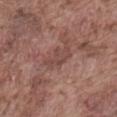Q: Is there a histopathology result?
A: catalogued during a skin exam; not biopsied
Q: Patient demographics?
A: male, in their mid-70s
Q: What is the lesion's diameter?
A: ≈4 mm
Q: Where on the body is the lesion?
A: the front of the torso
Q: What is the imaging modality?
A: ~15 mm tile from a whole-body skin photo
Q: What did automated image analysis measure?
A: a footprint of about 5.5 mm² and a shape-asymmetry score of about 0.4 (0 = symmetric); a lesion color around L≈45 a*≈20 b*≈23 in CIELAB, about 7 CIELAB-L* units darker than the surrounding skin, and a normalized border contrast of about 5; a border-irregularity rating of about 5/10 and internal color variation of about 1.5 on a 0–10 scale